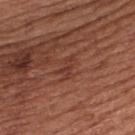No biopsy was performed on this lesion — it was imaged during a full skin examination and was not determined to be concerning.
A roughly 15 mm field-of-view crop from a total-body skin photograph.
The patient is a male in their mid- to late 60s.
Longest diameter approximately 3 mm.
The lesion is on the upper back.
This is a white-light tile.